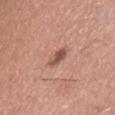Impression:
Recorded during total-body skin imaging; not selected for excision or biopsy.
Image and clinical context:
A 15 mm crop from a total-body photograph taken for skin-cancer surveillance. The lesion is located on the chest. A male subject, aged 43 to 47.